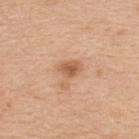biopsy status: no biopsy performed (imaged during a skin exam)
subject: female, about 30 years old
image source: ~15 mm crop, total-body skin-cancer survey
lighting: white-light
diameter: about 2.5 mm
anatomic site: the back